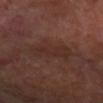Case summary:
- biopsy status · catalogued during a skin exam; not biopsied
- patient · female, in their mid- to late 50s
- diameter · about 5.5 mm
- imaging modality · ~15 mm tile from a whole-body skin photo
- anatomic site · the arm
- TBP lesion metrics · a footprint of about 10 mm², a shape eccentricity near 0.9, and a symmetry-axis asymmetry near 0.3; an average lesion color of about L≈30 a*≈19 b*≈23 (CIELAB) and a normalized lesion–skin contrast near 5; a border-irregularity index near 4.5/10 and peripheral color asymmetry of about 0.5; a classifier nevus-likeness of about 0/100 and lesion-presence confidence of about 100/100
- lighting · cross-polarized illumination